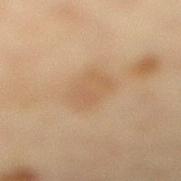Assessment:
Part of a total-body skin-imaging series; this lesion was reviewed on a skin check and was not flagged for biopsy.
Clinical summary:
The recorded lesion diameter is about 4 mm. A roughly 15 mm field-of-view crop from a total-body skin photograph. The tile uses cross-polarized illumination. The patient is a female aged 38 to 42. The lesion is on the right lower leg.